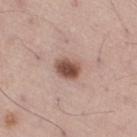biopsy_status: not biopsied; imaged during a skin examination
site: right thigh
lighting: white-light
automated_metrics:
  cielab_L: 51
  cielab_a: 20
  cielab_b: 25
  vs_skin_darker_L: 16.0
  vs_skin_contrast_norm: 10.5
  border_irregularity_0_10: 1.0
  color_variation_0_10: 4.0
  peripheral_color_asymmetry: 1.5
  nevus_likeness_0_100: 100
  lesion_detection_confidence_0_100: 100
lesion_size:
  long_diameter_mm_approx: 3.0
patient:
  sex: male
  age_approx: 55
image:
  source: total-body photography crop
  field_of_view_mm: 15